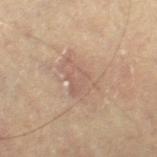Assessment: No biopsy was performed on this lesion — it was imaged during a full skin examination and was not determined to be concerning. Image and clinical context: The lesion is on the right thigh. A male subject, approximately 65 years of age. A close-up tile cropped from a whole-body skin photograph, about 15 mm across. Measured at roughly 5 mm in maximum diameter. Imaged with cross-polarized lighting. Automated tile analysis of the lesion measured a lesion area of about 11 mm² and an eccentricity of roughly 0.8. The analysis additionally found a lesion color around L≈52 a*≈15 b*≈24 in CIELAB and a lesion–skin lightness drop of about 6. The analysis additionally found a border-irregularity index near 4.5/10, a within-lesion color-variation index near 2.5/10, and peripheral color asymmetry of about 1.